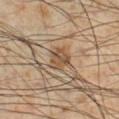notes = total-body-photography surveillance lesion; no biopsy.